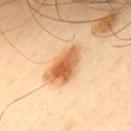The lesion was tiled from a total-body skin photograph and was not biopsied.
About 5.5 mm across.
This image is a 15 mm lesion crop taken from a total-body photograph.
The patient is a male roughly 50 years of age.
Located on the upper back.
The lesion-visualizer software estimated an average lesion color of about L≈64 a*≈26 b*≈43 (CIELAB) and a lesion–skin lightness drop of about 15. It also reported a border-irregularity index near 3/10 and a within-lesion color-variation index near 6.5/10.
This is a cross-polarized tile.